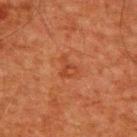Q: Was a biopsy performed?
A: catalogued during a skin exam; not biopsied
Q: What is the imaging modality?
A: ~15 mm crop, total-body skin-cancer survey
Q: Who is the patient?
A: male, aged around 60
Q: Lesion location?
A: the upper back